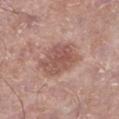Imaged during a routine full-body skin examination; the lesion was not biopsied and no histopathology is available. Imaged with white-light lighting. A region of skin cropped from a whole-body photographic capture, roughly 15 mm wide. A male patient, approximately 55 years of age. Located on the left lower leg. The total-body-photography lesion software estimated an eccentricity of roughly 0.5. The software also gave a lesion color around L≈54 a*≈22 b*≈25 in CIELAB, roughly 10 lightness units darker than nearby skin, and a lesion-to-skin contrast of about 7 (normalized; higher = more distinct). Longest diameter approximately 5 mm.